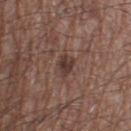{"biopsy_status": "not biopsied; imaged during a skin examination", "automated_metrics": {"border_irregularity_0_10": 2.5, "color_variation_0_10": 2.0, "peripheral_color_asymmetry": 1.0}, "image": {"source": "total-body photography crop", "field_of_view_mm": 15}, "site": "left thigh", "patient": {"sex": "male", "age_approx": 60}, "lesion_size": {"long_diameter_mm_approx": 2.5}}